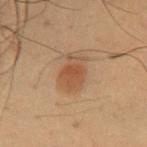| key | value |
|---|---|
| notes | imaged on a skin check; not biopsied |
| location | the chest |
| tile lighting | cross-polarized |
| subject | male, aged 48–52 |
| imaging modality | total-body-photography crop, ~15 mm field of view |
| size | ≈3.5 mm |
| automated lesion analysis | an average lesion color of about L≈40 a*≈17 b*≈28 (CIELAB) and about 7 CIELAB-L* units darker than the surrounding skin; internal color variation of about 2.5 on a 0–10 scale and peripheral color asymmetry of about 1; an automated nevus-likeness rating near 100 out of 100 and a detector confidence of about 100 out of 100 that the crop contains a lesion |